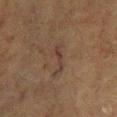Imaged during a routine full-body skin examination; the lesion was not biopsied and no histopathology is available. From the right lower leg. The tile uses cross-polarized illumination. A 15 mm close-up extracted from a 3D total-body photography capture. The subject is a male aged approximately 75.